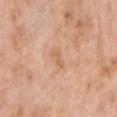{
  "biopsy_status": "not biopsied; imaged during a skin examination",
  "image": {
    "source": "total-body photography crop",
    "field_of_view_mm": 15
  },
  "site": "right upper arm",
  "patient": {
    "sex": "female",
    "age_approx": 70
  }
}